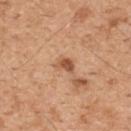Clinical impression:
This lesion was catalogued during total-body skin photography and was not selected for biopsy.
Clinical summary:
A male subject, in their mid- to late 40s. Cropped from a whole-body photographic skin survey; the tile spans about 15 mm. The lesion is on the mid back.